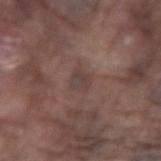Impression:
No biopsy was performed on this lesion — it was imaged during a full skin examination and was not determined to be concerning.
Context:
The lesion-visualizer software estimated a detector confidence of about 80 out of 100 that the crop contains a lesion. About 2.5 mm across. A male patient about 75 years old. Cropped from a total-body skin-imaging series; the visible field is about 15 mm. From the right forearm.